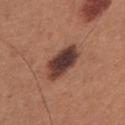Notes:
* notes — no biopsy performed (imaged during a skin exam)
* acquisition — 15 mm crop, total-body photography
* patient — male, aged 33 to 37
* body site — the chest